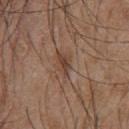Imaged during a routine full-body skin examination; the lesion was not biopsied and no histopathology is available. A 15 mm close-up extracted from a 3D total-body photography capture. The subject is a male about 55 years old. Automated image analysis of the tile measured a border-irregularity rating of about 3/10 and a color-variation rating of about 2/10. And it measured a nevus-likeness score of about 0/100 and a lesion-detection confidence of about 100/100. On the front of the torso.